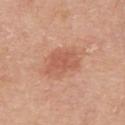Q: Is there a histopathology result?
A: catalogued during a skin exam; not biopsied
Q: Lesion size?
A: about 5 mm
Q: What lighting was used for the tile?
A: white-light
Q: Who is the patient?
A: male, aged around 80
Q: What is the imaging modality?
A: ~15 mm crop, total-body skin-cancer survey
Q: Where on the body is the lesion?
A: the upper back
Q: Automated lesion metrics?
A: an area of roughly 11 mm², an eccentricity of roughly 0.85, and two-axis asymmetry of about 0.3; about 9 CIELAB-L* units darker than the surrounding skin and a lesion-to-skin contrast of about 6 (normalized; higher = more distinct)